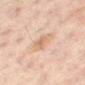The lesion was photographed on a routine skin check and not biopsied; there is no pathology result. A male patient roughly 60 years of age. A region of skin cropped from a whole-body photographic capture, roughly 15 mm wide. The lesion is on the mid back.